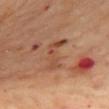{
  "biopsy_status": "not biopsied; imaged during a skin examination",
  "automated_metrics": {
    "area_mm2_approx": 8.0,
    "vs_skin_darker_L": 8.0
  },
  "lesion_size": {
    "long_diameter_mm_approx": 4.5
  },
  "site": "back",
  "patient": {
    "sex": "female",
    "age_approx": 55
  },
  "image": {
    "source": "total-body photography crop",
    "field_of_view_mm": 15
  },
  "lighting": "cross-polarized"
}